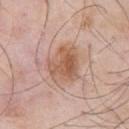| field | value |
|---|---|
| lighting | white-light illumination |
| patient | male, about 55 years old |
| image-analysis metrics | an area of roughly 12 mm², an eccentricity of roughly 0.4, and a shape-asymmetry score of about 0.25 (0 = symmetric); a mean CIELAB color near L≈57 a*≈21 b*≈31 and roughly 10 lightness units darker than nearby skin |
| anatomic site | the chest |
| lesion diameter | ~4 mm (longest diameter) |
| image source | ~15 mm tile from a whole-body skin photo |